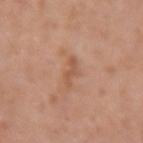biopsy_status: not biopsied; imaged during a skin examination
patient:
  sex: female
  age_approx: 40
lighting: white-light
image:
  source: total-body photography crop
  field_of_view_mm: 15
site: right upper arm